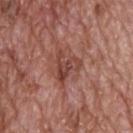{
  "biopsy_status": "not biopsied; imaged during a skin examination",
  "automated_metrics": {
    "cielab_L": 43,
    "cielab_a": 25,
    "cielab_b": 26,
    "vs_skin_darker_L": 9.0,
    "vs_skin_contrast_norm": 7.0,
    "nevus_likeness_0_100": 0,
    "lesion_detection_confidence_0_100": 95
  },
  "site": "upper back",
  "lighting": "white-light",
  "patient": {
    "sex": "male",
    "age_approx": 70
  },
  "image": {
    "source": "total-body photography crop",
    "field_of_view_mm": 15
  },
  "lesion_size": {
    "long_diameter_mm_approx": 3.5
  }
}